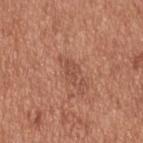Findings:
- biopsy status — catalogued during a skin exam; not biopsied
- TBP lesion metrics — a mean CIELAB color near L≈50 a*≈24 b*≈30; border irregularity of about 3 on a 0–10 scale and peripheral color asymmetry of about 0
- imaging modality — total-body-photography crop, ~15 mm field of view
- illumination — white-light
- patient — male, in their mid-50s
- diameter — ~2.5 mm (longest diameter)
- body site — the upper back